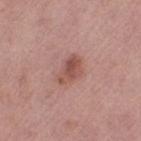follow-up = no biopsy performed (imaged during a skin exam)
image-analysis metrics = a lesion color around L≈52 a*≈24 b*≈25 in CIELAB and about 10 CIELAB-L* units darker than the surrounding skin; border irregularity of about 2.5 on a 0–10 scale and a peripheral color-asymmetry measure near 2
patient = female, approximately 65 years of age
lesion diameter = ~3.5 mm (longest diameter)
site = the right thigh
lighting = white-light
image source = 15 mm crop, total-body photography